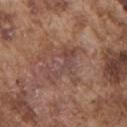Recorded during total-body skin imaging; not selected for excision or biopsy.
The patient is a male about 75 years old.
The lesion is on the chest.
A roughly 15 mm field-of-view crop from a total-body skin photograph.